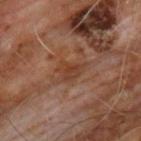Case summary:
* workup — imaged on a skin check; not biopsied